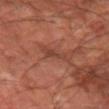Q: Was a biopsy performed?
A: catalogued during a skin exam; not biopsied
Q: What lighting was used for the tile?
A: cross-polarized illumination
Q: How was this image acquired?
A: ~15 mm crop, total-body skin-cancer survey
Q: Where on the body is the lesion?
A: the left forearm
Q: How large is the lesion?
A: ~3 mm (longest diameter)
Q: Patient demographics?
A: aged around 65